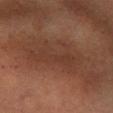| field | value |
|---|---|
| biopsy status | catalogued during a skin exam; not biopsied |
| imaging modality | 15 mm crop, total-body photography |
| subject | female, approximately 60 years of age |
| body site | the left forearm |